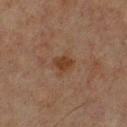Part of a total-body skin-imaging series; this lesion was reviewed on a skin check and was not flagged for biopsy. Captured under cross-polarized illumination. A 15 mm close-up extracted from a 3D total-body photography capture. The patient is a male in their mid- to late 40s. Measured at roughly 2.5 mm in maximum diameter. The lesion-visualizer software estimated a lesion area of about 4 mm², an outline eccentricity of about 0.65 (0 = round, 1 = elongated), and two-axis asymmetry of about 0.35. The analysis additionally found an average lesion color of about L≈31 a*≈17 b*≈26 (CIELAB), about 7 CIELAB-L* units darker than the surrounding skin, and a lesion-to-skin contrast of about 8 (normalized; higher = more distinct). The analysis additionally found a border-irregularity index near 3/10, internal color variation of about 1.5 on a 0–10 scale, and radial color variation of about 0.5. And it measured an automated nevus-likeness rating near 45 out of 100 and a lesion-detection confidence of about 100/100. The lesion is on the chest.